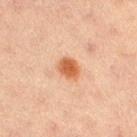Assessment:
Recorded during total-body skin imaging; not selected for excision or biopsy.
Background:
On the right thigh. Cropped from a total-body skin-imaging series; the visible field is about 15 mm. A female subject approximately 20 years of age.